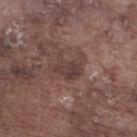Impression:
Recorded during total-body skin imaging; not selected for excision or biopsy.
Image and clinical context:
The lesion's longest dimension is about 3.5 mm. This is a white-light tile. From the right lower leg. The subject is a male aged around 75. Cropped from a whole-body photographic skin survey; the tile spans about 15 mm. The lesion-visualizer software estimated a mean CIELAB color near L≈39 a*≈17 b*≈20, about 7 CIELAB-L* units darker than the surrounding skin, and a normalized lesion–skin contrast near 6.5. The analysis additionally found a classifier nevus-likeness of about 5/100 and a detector confidence of about 100 out of 100 that the crop contains a lesion.